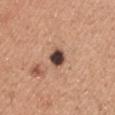Notes:
* biopsy status — no biopsy performed (imaged during a skin exam)
* diameter — ~2.5 mm (longest diameter)
* automated metrics — an eccentricity of roughly 0.45 and a symmetry-axis asymmetry near 0.2; an automated nevus-likeness rating near 15 out of 100 and lesion-presence confidence of about 100/100
* tile lighting — white-light
* patient — male, in their mid-40s
* image source — ~15 mm crop, total-body skin-cancer survey
* site — the right upper arm